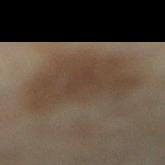Q: Was this lesion biopsied?
A: imaged on a skin check; not biopsied
Q: Patient demographics?
A: female, in their 60s
Q: What kind of image is this?
A: 15 mm crop, total-body photography
Q: Lesion size?
A: ~8 mm (longest diameter)
Q: Where on the body is the lesion?
A: the right lower leg